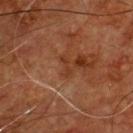This lesion was catalogued during total-body skin photography and was not selected for biopsy. A male subject, aged approximately 55. Located on the chest. The tile uses cross-polarized illumination. The recorded lesion diameter is about 2.5 mm. A close-up tile cropped from a whole-body skin photograph, about 15 mm across. An algorithmic analysis of the crop reported a lesion area of about 2 mm², a shape eccentricity near 0.95, and a shape-asymmetry score of about 0.45 (0 = symmetric).